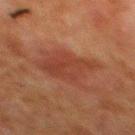  lesion_size:
    long_diameter_mm_approx: 6.0
  patient:
    sex: male
    age_approx: 80
  image:
    source: total-body photography crop
    field_of_view_mm: 15
  lighting: cross-polarized
  site: back
  automated_metrics:
    vs_skin_darker_L: 5.0
    vs_skin_contrast_norm: 5.5
    border_irregularity_0_10: 4.5
    color_variation_0_10: 2.5